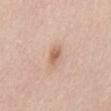follow-up: imaged on a skin check; not biopsied
lighting: white-light illumination
size: about 2.5 mm
body site: the front of the torso
acquisition: 15 mm crop, total-body photography
subject: female, aged around 30
automated metrics: a border-irregularity rating of about 2/10, a color-variation rating of about 3/10, and peripheral color asymmetry of about 1; a nevus-likeness score of about 70/100 and a detector confidence of about 100 out of 100 that the crop contains a lesion